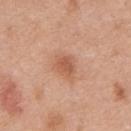Impression: This lesion was catalogued during total-body skin photography and was not selected for biopsy. Image and clinical context: Measured at roughly 3 mm in maximum diameter. From the chest. A male subject aged 68 to 72. Captured under white-light illumination. This image is a 15 mm lesion crop taken from a total-body photograph.